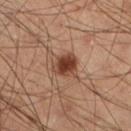Impression:
The lesion was tiled from a total-body skin photograph and was not biopsied.
Clinical summary:
The subject is a male aged 38 to 42. A roughly 15 mm field-of-view crop from a total-body skin photograph. The lesion is on the left lower leg. Measured at roughly 3.5 mm in maximum diameter. Automated image analysis of the tile measured a lesion color around L≈42 a*≈23 b*≈30 in CIELAB, a lesion–skin lightness drop of about 14, and a normalized border contrast of about 10.5.